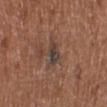No biopsy was performed on this lesion — it was imaged during a full skin examination and was not determined to be concerning. The patient is a male aged approximately 75. A region of skin cropped from a whole-body photographic capture, roughly 15 mm wide. Located on the leg.